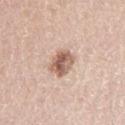The lesion was tiled from a total-body skin photograph and was not biopsied. Approximately 3.5 mm at its widest. A male subject aged 43 to 47. This is a white-light tile. Cropped from a whole-body photographic skin survey; the tile spans about 15 mm. From the right upper arm.